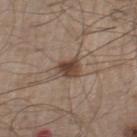The lesion was photographed on a routine skin check and not biopsied; there is no pathology result. The patient is a male aged 58–62. A 15 mm close-up tile from a total-body photography series done for melanoma screening. The lesion is located on the leg.